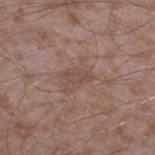Q: What are the patient's age and sex?
A: male, about 45 years old
Q: Lesion location?
A: the right thigh
Q: What kind of image is this?
A: ~15 mm crop, total-body skin-cancer survey
Q: Lesion size?
A: ~3 mm (longest diameter)
Q: Illumination type?
A: white-light illumination